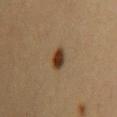| feature | finding |
|---|---|
| diameter | about 2.5 mm |
| patient | female, roughly 30 years of age |
| tile lighting | cross-polarized |
| anatomic site | the left upper arm |
| automated metrics | a shape eccentricity near 0.45; a lesion color around L≈35 a*≈16 b*≈29 in CIELAB, roughly 13 lightness units darker than nearby skin, and a normalized border contrast of about 11.5; a border-irregularity rating of about 1.5/10, a color-variation rating of about 6/10, and peripheral color asymmetry of about 2 |
| image | 15 mm crop, total-body photography |